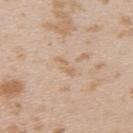The lesion was photographed on a routine skin check and not biopsied; there is no pathology result.
A 15 mm close-up extracted from a 3D total-body photography capture.
Automated tile analysis of the lesion measured a footprint of about 2.5 mm², an eccentricity of roughly 0.9, and two-axis asymmetry of about 0.4. The analysis additionally found a border-irregularity rating of about 5/10, a color-variation rating of about 0/10, and a peripheral color-asymmetry measure near 0.
A female patient, in their mid- to late 20s.
The lesion's longest dimension is about 3 mm.
Imaged with white-light lighting.
The lesion is located on the upper back.